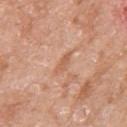The lesion was tiled from a total-body skin photograph and was not biopsied. A region of skin cropped from a whole-body photographic capture, roughly 15 mm wide. Located on the right upper arm. Measured at roughly 3 mm in maximum diameter. The subject is a female roughly 75 years of age.